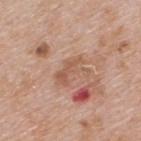The lesion was tiled from a total-body skin photograph and was not biopsied.
A region of skin cropped from a whole-body photographic capture, roughly 15 mm wide.
Automated tile analysis of the lesion measured a shape eccentricity near 0.8 and two-axis asymmetry of about 0.45. It also reported about 7 CIELAB-L* units darker than the surrounding skin and a normalized lesion–skin contrast near 5.5.
Located on the upper back.
A male patient, in their mid- to late 60s.
Longest diameter approximately 4 mm.